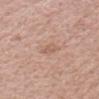Impression:
Recorded during total-body skin imaging; not selected for excision or biopsy.
Acquisition and patient details:
A 15 mm close-up tile from a total-body photography series done for melanoma screening. Imaged with white-light lighting. The subject is a male aged around 45. Automated image analysis of the tile measured a footprint of about 3 mm² and an eccentricity of roughly 0.9. It also reported an average lesion color of about L≈60 a*≈19 b*≈29 (CIELAB), a lesion–skin lightness drop of about 7, and a normalized lesion–skin contrast near 5. The lesion is on the head or neck.